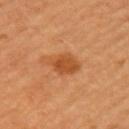Longest diameter approximately 3.5 mm. Imaged with cross-polarized lighting. A 15 mm crop from a total-body photograph taken for skin-cancer surveillance. The lesion is located on the arm. A female patient about 45 years old.